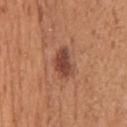follow-up: imaged on a skin check; not biopsied | acquisition: ~15 mm crop, total-body skin-cancer survey | anatomic site: the chest | subject: male, aged 53–57 | size: ≈4 mm | illumination: white-light illumination | automated metrics: a footprint of about 7 mm², an outline eccentricity of about 0.8 (0 = round, 1 = elongated), and a shape-asymmetry score of about 0.3 (0 = symmetric); a border-irregularity index near 3/10, internal color variation of about 3 on a 0–10 scale, and a peripheral color-asymmetry measure near 1; a detector confidence of about 100 out of 100 that the crop contains a lesion.